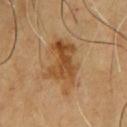follow-up=no biopsy performed (imaged during a skin exam); anatomic site=the chest; subject=male, aged 63–67; imaging modality=total-body-photography crop, ~15 mm field of view.